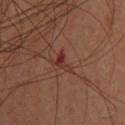follow-up=catalogued during a skin exam; not biopsied
patient=female, about 45 years old
imaging modality=~15 mm crop, total-body skin-cancer survey
location=the back